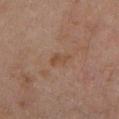{
  "biopsy_status": "not biopsied; imaged during a skin examination",
  "lighting": "cross-polarized",
  "patient": {
    "sex": "female",
    "age_approx": 60
  },
  "image": {
    "source": "total-body photography crop",
    "field_of_view_mm": 15
  },
  "site": "left lower leg",
  "automated_metrics": {
    "cielab_L": 43,
    "cielab_a": 17,
    "cielab_b": 28,
    "vs_skin_darker_L": 5.0,
    "vs_skin_contrast_norm": 6.0,
    "border_irregularity_0_10": 4.0,
    "color_variation_0_10": 1.0
  }
}